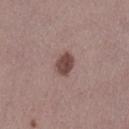A close-up tile cropped from a whole-body skin photograph, about 15 mm across. A female patient roughly 40 years of age. Imaged with white-light lighting. The lesion-visualizer software estimated a mean CIELAB color near L≈44 a*≈19 b*≈20, a lesion–skin lightness drop of about 14, and a normalized lesion–skin contrast near 10.5. It also reported an automated nevus-likeness rating near 70 out of 100. The lesion's longest dimension is about 2.5 mm. The lesion is on the lower back.